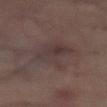Q: Is there a histopathology result?
A: imaged on a skin check; not biopsied
Q: What kind of image is this?
A: ~15 mm crop, total-body skin-cancer survey
Q: How large is the lesion?
A: about 6.5 mm
Q: What did automated image analysis measure?
A: an eccentricity of roughly 0.8 and two-axis asymmetry of about 0.35; a within-lesion color-variation index near 3.5/10 and radial color variation of about 1
Q: Patient demographics?
A: male, aged 58–62
Q: Lesion location?
A: the abdomen
Q: How was the tile lit?
A: cross-polarized